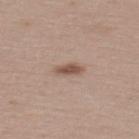Imaged during a routine full-body skin examination; the lesion was not biopsied and no histopathology is available.
Located on the upper back.
A region of skin cropped from a whole-body photographic capture, roughly 15 mm wide.
A female subject, in their mid- to late 50s.
Automated image analysis of the tile measured an average lesion color of about L≈52 a*≈18 b*≈26 (CIELAB) and a lesion–skin lightness drop of about 12. And it measured a lesion-detection confidence of about 100/100.
Approximately 3 mm at its widest.